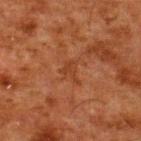No biopsy was performed on this lesion — it was imaged during a full skin examination and was not determined to be concerning. This is a cross-polarized tile. Cropped from a total-body skin-imaging series; the visible field is about 15 mm. The recorded lesion diameter is about 2.5 mm. The subject is a male aged 58–62. From the back.